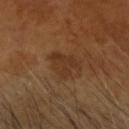* workup: total-body-photography surveillance lesion; no biopsy
* automated metrics: a mean CIELAB color near L≈34 a*≈19 b*≈31 and about 6 CIELAB-L* units darker than the surrounding skin
* body site: the head or neck
* patient: male, aged approximately 65
* acquisition: ~15 mm crop, total-body skin-cancer survey
* lesion size: about 4 mm